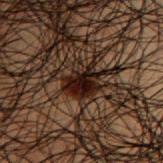Imaged during a routine full-body skin examination; the lesion was not biopsied and no histopathology is available. Longest diameter approximately 3.5 mm. Automated image analysis of the tile measured a footprint of about 9.5 mm², an eccentricity of roughly 0.35, and a shape-asymmetry score of about 0.3 (0 = symmetric). The software also gave an average lesion color of about L≈13 a*≈13 b*≈15 (CIELAB) and roughly 10 lightness units darker than nearby skin. And it measured border irregularity of about 3.5 on a 0–10 scale, a color-variation rating of about 5/10, and a peripheral color-asymmetry measure near 1.5. It also reported a classifier nevus-likeness of about 100/100. The lesion is located on the upper back. Cropped from a total-body skin-imaging series; the visible field is about 15 mm. This is a cross-polarized tile. A male subject, aged approximately 50.